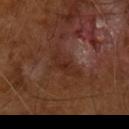{"biopsy_status": "not biopsied; imaged during a skin examination"}